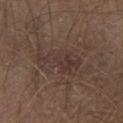Part of a total-body skin-imaging series; this lesion was reviewed on a skin check and was not flagged for biopsy.
A male patient roughly 65 years of age.
The lesion is located on the left thigh.
This image is a 15 mm lesion crop taken from a total-body photograph.